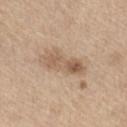Imaged during a routine full-body skin examination; the lesion was not biopsied and no histopathology is available. The subject is a male about 70 years old. A region of skin cropped from a whole-body photographic capture, roughly 15 mm wide. On the leg.